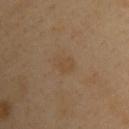Case summary:
– notes · catalogued during a skin exam; not biopsied
– automated metrics · a mean CIELAB color near L≈46 a*≈15 b*≈31 and a lesion-to-skin contrast of about 4.5 (normalized; higher = more distinct); a border-irregularity rating of about 2/10 and a peripheral color-asymmetry measure near 0.5
– body site · the left upper arm
– image source · 15 mm crop, total-body photography
– diameter · ~2.5 mm (longest diameter)
– tile lighting · cross-polarized
– subject · male, roughly 40 years of age